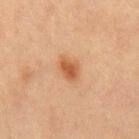Clinical impression: Recorded during total-body skin imaging; not selected for excision or biopsy. Background: A female patient, roughly 70 years of age. Measured at roughly 3.5 mm in maximum diameter. The lesion is located on the right thigh. Cropped from a whole-body photographic skin survey; the tile spans about 15 mm. The total-body-photography lesion software estimated a lesion color around L≈50 a*≈22 b*≈35 in CIELAB, a lesion–skin lightness drop of about 10, and a normalized border contrast of about 8. And it measured a border-irregularity index near 2.5/10, a color-variation rating of about 3/10, and a peripheral color-asymmetry measure near 1. It also reported a nevus-likeness score of about 95/100.